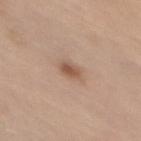Recorded during total-body skin imaging; not selected for excision or biopsy.
From the chest.
A female subject, approximately 65 years of age.
The lesion's longest dimension is about 2.5 mm.
A 15 mm crop from a total-body photograph taken for skin-cancer surveillance.